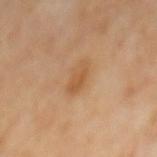Notes:
- workup · imaged on a skin check; not biopsied
- image · ~15 mm crop, total-body skin-cancer survey
- size · ~3.5 mm (longest diameter)
- subject · female, approximately 50 years of age
- anatomic site · the back